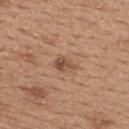Q: How was the tile lit?
A: white-light illumination
Q: How was this image acquired?
A: ~15 mm tile from a whole-body skin photo
Q: Lesion location?
A: the upper back
Q: What did automated image analysis measure?
A: an area of roughly 4 mm² and an outline eccentricity of about 0.75 (0 = round, 1 = elongated)
Q: Who is the patient?
A: female, in their mid- to late 30s
Q: How large is the lesion?
A: about 3 mm